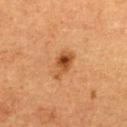Clinical impression: Recorded during total-body skin imaging; not selected for excision or biopsy. Acquisition and patient details: A 15 mm crop from a total-body photograph taken for skin-cancer surveillance. A female subject, aged around 40. Longest diameter approximately 3.5 mm. This is a cross-polarized tile. On the upper back. The total-body-photography lesion software estimated a lesion area of about 5 mm² and two-axis asymmetry of about 0.4. The software also gave a within-lesion color-variation index near 6/10 and peripheral color asymmetry of about 2.